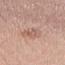Q: Was a biopsy performed?
A: no biopsy performed (imaged during a skin exam)
Q: How large is the lesion?
A: ~3 mm (longest diameter)
Q: How was this image acquired?
A: ~15 mm crop, total-body skin-cancer survey
Q: What did automated image analysis measure?
A: a lesion area of about 3 mm², a shape eccentricity near 0.9, and a shape-asymmetry score of about 0.45 (0 = symmetric); a lesion color around L≈59 a*≈21 b*≈27 in CIELAB; border irregularity of about 6.5 on a 0–10 scale and radial color variation of about 0; a classifier nevus-likeness of about 0/100 and lesion-presence confidence of about 100/100
Q: Lesion location?
A: the left thigh
Q: How was the tile lit?
A: white-light illumination
Q: Patient demographics?
A: male, about 65 years old